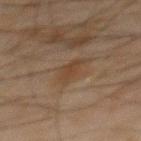A lesion tile, about 15 mm wide, cut from a 3D total-body photograph. Measured at roughly 3.5 mm in maximum diameter. On the mid back. A male subject aged approximately 45. The lesion-visualizer software estimated a classifier nevus-likeness of about 10/100 and a detector confidence of about 100 out of 100 that the crop contains a lesion. The tile uses cross-polarized illumination.